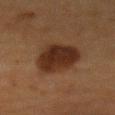Captured during whole-body skin photography for melanoma surveillance; the lesion was not biopsied.
Imaged with cross-polarized lighting.
A roughly 15 mm field-of-view crop from a total-body skin photograph.
The total-body-photography lesion software estimated a shape-asymmetry score of about 0.2 (0 = symmetric). The software also gave border irregularity of about 2.5 on a 0–10 scale, a within-lesion color-variation index near 4/10, and peripheral color asymmetry of about 1. And it measured a nevus-likeness score of about 95/100 and lesion-presence confidence of about 100/100.
The lesion is located on the mid back.
A female patient approximately 50 years of age.
About 7 mm across.